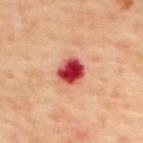Assessment: This lesion was catalogued during total-body skin photography and was not selected for biopsy. Background: The subject is a male aged approximately 65. On the upper back. The tile uses cross-polarized illumination. A 15 mm crop from a total-body photograph taken for skin-cancer surveillance. Measured at roughly 3 mm in maximum diameter.